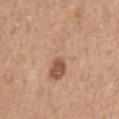  biopsy_status: not biopsied; imaged during a skin examination
  automated_metrics:
    area_mm2_approx: 16.0
    eccentricity: 0.85
    shape_asymmetry: 0.5
  image:
    source: total-body photography crop
    field_of_view_mm: 15
  site: right upper arm
  patient:
    sex: male
    age_approx: 50
  lesion_size:
    long_diameter_mm_approx: 6.5
  lighting: white-light